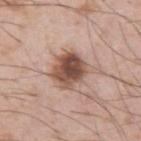The lesion was tiled from a total-body skin photograph and was not biopsied.
Captured under white-light illumination.
A 15 mm crop from a total-body photograph taken for skin-cancer surveillance.
Automated tile analysis of the lesion measured an area of roughly 14 mm², an outline eccentricity of about 0.45 (0 = round, 1 = elongated), and a shape-asymmetry score of about 0.3 (0 = symmetric). And it measured a mean CIELAB color near L≈52 a*≈19 b*≈26 and roughly 15 lightness units darker than nearby skin. It also reported an automated nevus-likeness rating near 90 out of 100 and lesion-presence confidence of about 100/100.
A male subject, aged around 55.
Approximately 5 mm at its widest.